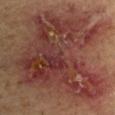Q: Was a biopsy performed?
A: catalogued during a skin exam; not biopsied
Q: What are the patient's age and sex?
A: male, aged 63–67
Q: What lighting was used for the tile?
A: cross-polarized illumination
Q: What is the imaging modality?
A: 15 mm crop, total-body photography
Q: How large is the lesion?
A: ~11.5 mm (longest diameter)
Q: Lesion location?
A: the arm